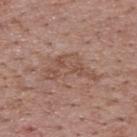| field | value |
|---|---|
| biopsy status | no biopsy performed (imaged during a skin exam) |
| patient | male, aged around 70 |
| anatomic site | the upper back |
| tile lighting | white-light illumination |
| lesion diameter | ≈6.5 mm |
| acquisition | total-body-photography crop, ~15 mm field of view |
| automated lesion analysis | a border-irregularity rating of about 7.5/10, a color-variation rating of about 3.5/10, and peripheral color asymmetry of about 1 |